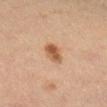Q: Was this lesion biopsied?
A: no biopsy performed (imaged during a skin exam)
Q: Who is the patient?
A: female, roughly 30 years of age
Q: How was the tile lit?
A: cross-polarized
Q: What is the anatomic site?
A: the right lower leg
Q: How was this image acquired?
A: ~15 mm tile from a whole-body skin photo